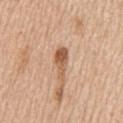Q: What is the anatomic site?
A: the abdomen
Q: What kind of image is this?
A: 15 mm crop, total-body photography
Q: What are the patient's age and sex?
A: male, aged 68 to 72